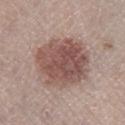Clinical impression:
Captured during whole-body skin photography for melanoma surveillance; the lesion was not biopsied.
Background:
The lesion's longest dimension is about 6.5 mm. A close-up tile cropped from a whole-body skin photograph, about 15 mm across. The lesion is on the right lower leg. An algorithmic analysis of the crop reported an area of roughly 30 mm² and an outline eccentricity of about 0.4 (0 = round, 1 = elongated). The software also gave a classifier nevus-likeness of about 45/100 and a lesion-detection confidence of about 100/100. The patient is a male about 55 years old.